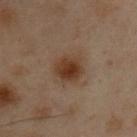Impression:
No biopsy was performed on this lesion — it was imaged during a full skin examination and was not determined to be concerning.
Clinical summary:
Automated tile analysis of the lesion measured a footprint of about 8.5 mm², a shape eccentricity near 0.5, and two-axis asymmetry of about 0.2. The software also gave a border-irregularity index near 2/10, a color-variation rating of about 3.5/10, and a peripheral color-asymmetry measure near 1. And it measured a classifier nevus-likeness of about 100/100 and lesion-presence confidence of about 100/100. This image is a 15 mm lesion crop taken from a total-body photograph. Measured at roughly 3.5 mm in maximum diameter. The subject is a male roughly 55 years of age. The lesion is on the chest. The tile uses cross-polarized illumination.